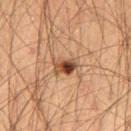Captured during whole-body skin photography for melanoma surveillance; the lesion was not biopsied. A male patient aged 63–67. From the leg. Captured under cross-polarized illumination. Longest diameter approximately 3 mm. A roughly 15 mm field-of-view crop from a total-body skin photograph. The total-body-photography lesion software estimated an eccentricity of roughly 0.75 and two-axis asymmetry of about 0.25. It also reported a border-irregularity index near 3/10 and internal color variation of about 10 on a 0–10 scale. It also reported a nevus-likeness score of about 95/100 and a detector confidence of about 100 out of 100 that the crop contains a lesion.